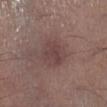Case summary:
• workup: no biopsy performed (imaged during a skin exam)
• anatomic site: the leg
• imaging modality: total-body-photography crop, ~15 mm field of view
• patient: male, aged 53–57